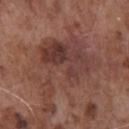– biopsy status — catalogued during a skin exam; not biopsied
– patient — male, aged 73 to 77
– image-analysis metrics — an area of roughly 36 mm² and a shape-asymmetry score of about 0.55 (0 = symmetric); a normalized lesion–skin contrast near 7; a classifier nevus-likeness of about 0/100 and lesion-presence confidence of about 80/100
– image source — ~15 mm crop, total-body skin-cancer survey
– diameter — ~10.5 mm (longest diameter)
– illumination — white-light illumination
– body site — the chest